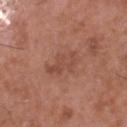Imaged during a routine full-body skin examination; the lesion was not biopsied and no histopathology is available. This image is a 15 mm lesion crop taken from a total-body photograph. Measured at roughly 4.5 mm in maximum diameter. On the head or neck. A male subject, approximately 50 years of age. An algorithmic analysis of the crop reported an average lesion color of about L≈48 a*≈24 b*≈28 (CIELAB), roughly 7 lightness units darker than nearby skin, and a lesion-to-skin contrast of about 5 (normalized; higher = more distinct). The tile uses white-light illumination.